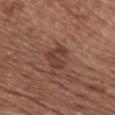biopsy status: no biopsy performed (imaged during a skin exam)
automated lesion analysis: about 8 CIELAB-L* units darker than the surrounding skin; border irregularity of about 3.5 on a 0–10 scale, a color-variation rating of about 3/10, and a peripheral color-asymmetry measure near 1; a lesion-detection confidence of about 100/100
lighting: white-light
image source: ~15 mm crop, total-body skin-cancer survey
size: about 3 mm
location: the chest
patient: female, aged approximately 75